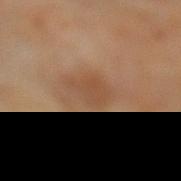Clinical impression:
The lesion was photographed on a routine skin check and not biopsied; there is no pathology result.
Context:
About 4 mm across. The lesion is on the right leg. Captured under cross-polarized illumination. The total-body-photography lesion software estimated a footprint of about 8 mm², an outline eccentricity of about 0.7 (0 = round, 1 = elongated), and a shape-asymmetry score of about 0.3 (0 = symmetric). It also reported a mean CIELAB color near L≈47 a*≈19 b*≈31 and a lesion–skin lightness drop of about 6. The analysis additionally found a nevus-likeness score of about 0/100 and a detector confidence of about 100 out of 100 that the crop contains a lesion. A roughly 15 mm field-of-view crop from a total-body skin photograph. The patient is a female aged 63 to 67.